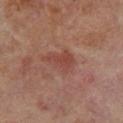* workup: catalogued during a skin exam; not biopsied
* subject: male, roughly 70 years of age
* automated lesion analysis: a footprint of about 7.5 mm², an eccentricity of roughly 0.8, and a shape-asymmetry score of about 0.4 (0 = symmetric); border irregularity of about 5 on a 0–10 scale and a peripheral color-asymmetry measure near 1
* image: 15 mm crop, total-body photography
* size: ≈4 mm
* site: the right lower leg
* tile lighting: cross-polarized illumination